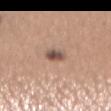Located on the abdomen. Measured at roughly 2.5 mm in maximum diameter. A close-up tile cropped from a whole-body skin photograph, about 15 mm across. The patient is a female in their 40s. Imaged with white-light lighting.